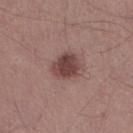Clinical impression: Imaged during a routine full-body skin examination; the lesion was not biopsied and no histopathology is available. Clinical summary: A male subject, in their mid-40s. The tile uses white-light illumination. The lesion is located on the right lower leg. A roughly 15 mm field-of-view crop from a total-body skin photograph.